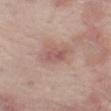| key | value |
|---|---|
| workup | no biopsy performed (imaged during a skin exam) |
| image source | ~15 mm crop, total-body skin-cancer survey |
| anatomic site | the left thigh |
| size | ~3.5 mm (longest diameter) |
| patient | male, aged 63 to 67 |